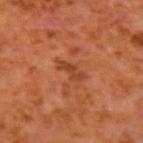Impression: The lesion was photographed on a routine skin check and not biopsied; there is no pathology result. Background: A lesion tile, about 15 mm wide, cut from a 3D total-body photograph. The lesion's longest dimension is about 3 mm. Automated image analysis of the tile measured a footprint of about 3.5 mm² and two-axis asymmetry of about 0.45. The analysis additionally found a mean CIELAB color near L≈41 a*≈27 b*≈35 and a normalized lesion–skin contrast near 6.5. The tile uses cross-polarized illumination. The lesion is located on the left upper arm. The patient is a male about 60 years old.